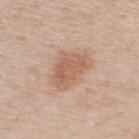patient:
  sex: male
  age_approx: 60
image:
  source: total-body photography crop
  field_of_view_mm: 15
lesion_size:
  long_diameter_mm_approx: 5.0
site: back
lighting: white-light